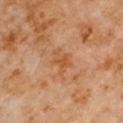Notes:
* biopsy status · imaged on a skin check; not biopsied
* automated lesion analysis · border irregularity of about 5.5 on a 0–10 scale, a within-lesion color-variation index near 3/10, and peripheral color asymmetry of about 1
* site · the arm
* lighting · cross-polarized illumination
* subject · male, approximately 60 years of age
* lesion size · ≈3.5 mm
* imaging modality · 15 mm crop, total-body photography